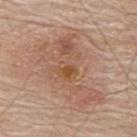notes: total-body-photography surveillance lesion; no biopsy
anatomic site: the upper back
subject: male, aged 68 to 72
illumination: white-light illumination
image-analysis metrics: a lesion area of about 28 mm², a shape eccentricity near 0.85, and a symmetry-axis asymmetry near 0.55; a mean CIELAB color near L≈55 a*≈19 b*≈30 and a lesion-to-skin contrast of about 6 (normalized; higher = more distinct); a border-irregularity rating of about 8/10, a color-variation rating of about 6/10, and a peripheral color-asymmetry measure near 1.5; an automated nevus-likeness rating near 10 out of 100 and a detector confidence of about 100 out of 100 that the crop contains a lesion
image source: ~15 mm tile from a whole-body skin photo
diameter: ≈8.5 mm